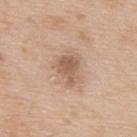This is a white-light tile. From the upper back. The recorded lesion diameter is about 3.5 mm. Automated image analysis of the tile measured a footprint of about 6.5 mm² and two-axis asymmetry of about 0.35. It also reported about 11 CIELAB-L* units darker than the surrounding skin and a lesion-to-skin contrast of about 7 (normalized; higher = more distinct). It also reported a border-irregularity index near 3.5/10, a within-lesion color-variation index near 3/10, and peripheral color asymmetry of about 1. The software also gave a classifier nevus-likeness of about 10/100 and a lesion-detection confidence of about 100/100. The subject is a male aged approximately 65. A 15 mm close-up extracted from a 3D total-body photography capture.